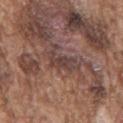The lesion was tiled from a total-body skin photograph and was not biopsied.
The tile uses white-light illumination.
A 15 mm close-up tile from a total-body photography series done for melanoma screening.
The lesion is located on the mid back.
The recorded lesion diameter is about 3.5 mm.
The patient is a male approximately 75 years of age.